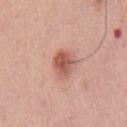| field | value |
|---|---|
| notes | no biopsy performed (imaged during a skin exam) |
| tile lighting | white-light |
| lesion size | about 3 mm |
| image | ~15 mm tile from a whole-body skin photo |
| patient | male, aged 53–57 |
| automated lesion analysis | a color-variation rating of about 4.5/10 and radial color variation of about 1.5; a classifier nevus-likeness of about 95/100 and a lesion-detection confidence of about 100/100 |
| location | the chest |